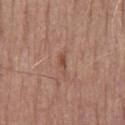Part of a total-body skin-imaging series; this lesion was reviewed on a skin check and was not flagged for biopsy. From the mid back. A male patient, about 80 years old. Automated tile analysis of the lesion measured a lesion color around L≈49 a*≈21 b*≈28 in CIELAB, about 8 CIELAB-L* units darker than the surrounding skin, and a normalized border contrast of about 6. And it measured a border-irregularity rating of about 4/10. A region of skin cropped from a whole-body photographic capture, roughly 15 mm wide.